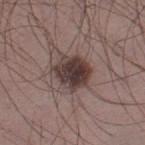* notes: imaged on a skin check; not biopsied
* site: the leg
* lighting: white-light illumination
* lesion diameter: about 4 mm
* subject: male, approximately 35 years of age
* imaging modality: ~15 mm crop, total-body skin-cancer survey